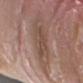notes = total-body-photography surveillance lesion; no biopsy | location = the arm | lighting = white-light | patient = male, in their 40s | diameter = ≈4.5 mm | image = total-body-photography crop, ~15 mm field of view.